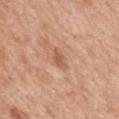No biopsy was performed on this lesion — it was imaged during a full skin examination and was not determined to be concerning.
Cropped from a total-body skin-imaging series; the visible field is about 15 mm.
Located on the mid back.
The patient is a female about 40 years old.